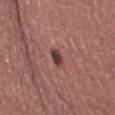This is a white-light tile.
A roughly 15 mm field-of-view crop from a total-body skin photograph.
A female patient, aged around 60.
Automated tile analysis of the lesion measured a lesion area of about 3 mm², an eccentricity of roughly 0.85, and a symmetry-axis asymmetry near 0.2. It also reported a lesion color around L≈39 a*≈21 b*≈19 in CIELAB, a lesion–skin lightness drop of about 13, and a normalized border contrast of about 10.5. And it measured peripheral color asymmetry of about 1.5. It also reported a nevus-likeness score of about 65/100.
The lesion is located on the left thigh.
The recorded lesion diameter is about 2.5 mm.